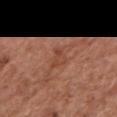Captured during whole-body skin photography for melanoma surveillance; the lesion was not biopsied. Imaged with white-light lighting. On the chest. The patient is a female roughly 75 years of age. A close-up tile cropped from a whole-body skin photograph, about 15 mm across. Longest diameter approximately 2.5 mm.